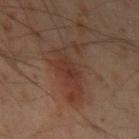The lesion was photographed on a routine skin check and not biopsied; there is no pathology result.
From the left upper arm.
A lesion tile, about 15 mm wide, cut from a 3D total-body photograph.
A male patient, aged approximately 50.
The lesion's longest dimension is about 7 mm.
Imaged with cross-polarized lighting.